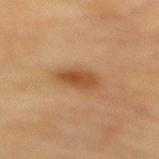No biopsy was performed on this lesion — it was imaged during a full skin examination and was not determined to be concerning.
From the mid back.
A 15 mm crop from a total-body photograph taken for skin-cancer surveillance.
Captured under cross-polarized illumination.
The subject is a female approximately 80 years of age.
Measured at roughly 4 mm in maximum diameter.